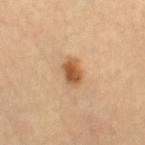Assessment: Part of a total-body skin-imaging series; this lesion was reviewed on a skin check and was not flagged for biopsy.